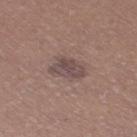Captured during whole-body skin photography for melanoma surveillance; the lesion was not biopsied.
A female patient, aged 38 to 42.
On the right thigh.
Longest diameter approximately 4 mm.
An algorithmic analysis of the crop reported an eccentricity of roughly 0.8 and a shape-asymmetry score of about 0.3 (0 = symmetric).
Cropped from a whole-body photographic skin survey; the tile spans about 15 mm.
Imaged with white-light lighting.